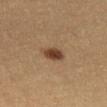Case summary:
- biopsy status: no biopsy performed (imaged during a skin exam)
- subject: female, aged approximately 30
- image: ~15 mm crop, total-body skin-cancer survey
- anatomic site: the left thigh